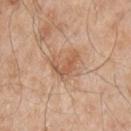| key | value |
|---|---|
| biopsy status | total-body-photography surveillance lesion; no biopsy |
| lesion size | ≈3.5 mm |
| automated lesion analysis | an area of roughly 6.5 mm²; a lesion color around L≈58 a*≈21 b*≈33 in CIELAB and a lesion–skin lightness drop of about 9; a border-irregularity index near 5.5/10, a within-lesion color-variation index near 3/10, and radial color variation of about 1 |
| subject | male, roughly 65 years of age |
| image | 15 mm crop, total-body photography |
| body site | the arm |
| illumination | white-light illumination |